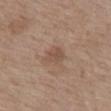This lesion was catalogued during total-body skin photography and was not selected for biopsy.
Cropped from a whole-body photographic skin survey; the tile spans about 15 mm.
Measured at roughly 2.5 mm in maximum diameter.
The total-body-photography lesion software estimated a lesion color around L≈50 a*≈17 b*≈27 in CIELAB and a lesion–skin lightness drop of about 7. It also reported an automated nevus-likeness rating near 0 out of 100.
The subject is a female aged 68–72.
Imaged with white-light lighting.
The lesion is on the mid back.